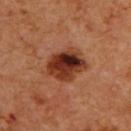Case summary:
* biopsy status — imaged on a skin check; not biopsied
* illumination — cross-polarized
* subject — male, aged 58–62
* TBP lesion metrics — a border-irregularity index near 2.5/10, internal color variation of about 10 on a 0–10 scale, and peripheral color asymmetry of about 4; a nevus-likeness score of about 85/100 and a detector confidence of about 100 out of 100 that the crop contains a lesion
* location — the back
* diameter — ~5.5 mm (longest diameter)
* image — ~15 mm tile from a whole-body skin photo